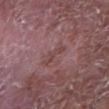biopsy_status: not biopsied; imaged during a skin examination
lesion_size:
  long_diameter_mm_approx: 3.0
lighting: white-light
image:
  source: total-body photography crop
  field_of_view_mm: 15
patient:
  sex: male
  age_approx: 65
site: right forearm